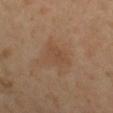– illumination: cross-polarized
– site: the right lower leg
– patient: female, about 50 years old
– image-analysis metrics: a lesion color around L≈48 a*≈18 b*≈31 in CIELAB, about 5 CIELAB-L* units darker than the surrounding skin, and a lesion-to-skin contrast of about 4.5 (normalized; higher = more distinct); a border-irregularity index near 3/10, a within-lesion color-variation index near 2/10, and radial color variation of about 0.5; a classifier nevus-likeness of about 0/100 and a lesion-detection confidence of about 100/100
– image source: ~15 mm crop, total-body skin-cancer survey